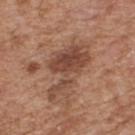notes: imaged on a skin check; not biopsied
image: 15 mm crop, total-body photography
patient: male, about 65 years old
tile lighting: white-light illumination
diameter: ~7 mm (longest diameter)
site: the upper back